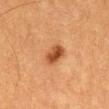Assessment:
The lesion was photographed on a routine skin check and not biopsied; there is no pathology result.
Background:
Automated image analysis of the tile measured a normalized border contrast of about 9.5. It also reported an automated nevus-likeness rating near 95 out of 100 and a lesion-detection confidence of about 100/100. About 3 mm across. A male patient aged 58–62. A close-up tile cropped from a whole-body skin photograph, about 15 mm across. This is a cross-polarized tile. The lesion is on the abdomen.